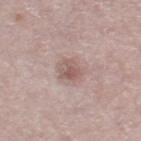biopsy_status: not biopsied; imaged during a skin examination
site: leg
lesion_size:
  long_diameter_mm_approx: 2.5
image:
  source: total-body photography crop
  field_of_view_mm: 15
patient:
  sex: female
  age_approx: 65
lighting: white-light